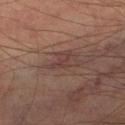Case summary:
• biopsy status: no biopsy performed (imaged during a skin exam)
• site: the leg
• subject: male, in their mid-70s
• image: ~15 mm tile from a whole-body skin photo
• lesion diameter: about 2.5 mm
• image-analysis metrics: roughly 5 lightness units darker than nearby skin and a normalized border contrast of about 5.5; a border-irregularity rating of about 5.5/10, a within-lesion color-variation index near 0/10, and peripheral color asymmetry of about 0; a nevus-likeness score of about 0/100 and a lesion-detection confidence of about 65/100